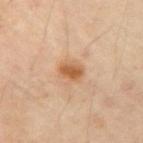Image and clinical context:
A 15 mm close-up tile from a total-body photography series done for melanoma screening. On the right upper arm. The subject is a female about 45 years old.